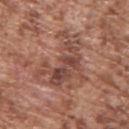The lesion was tiled from a total-body skin photograph and was not biopsied. The total-body-photography lesion software estimated an average lesion color of about L≈47 a*≈22 b*≈26 (CIELAB), about 9 CIELAB-L* units darker than the surrounding skin, and a normalized border contrast of about 7. It also reported a border-irregularity rating of about 9.5/10 and radial color variation of about 2.5. From the upper back. A male patient, roughly 75 years of age. A 15 mm close-up tile from a total-body photography series done for melanoma screening. Captured under white-light illumination.